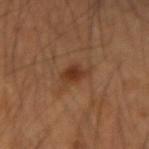Clinical impression:
Part of a total-body skin-imaging series; this lesion was reviewed on a skin check and was not flagged for biopsy.
Image and clinical context:
The lesion-visualizer software estimated a footprint of about 4.5 mm², a shape eccentricity near 0.65, and a symmetry-axis asymmetry near 0.3. The analysis additionally found a color-variation rating of about 2.5/10 and peripheral color asymmetry of about 0.5. The lesion is on the arm. A 15 mm crop from a total-body photograph taken for skin-cancer surveillance. Longest diameter approximately 2.5 mm. A male patient, about 50 years old. Imaged with cross-polarized lighting.